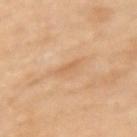patient — female, aged around 60 | acquisition — ~15 mm tile from a whole-body skin photo | location — the arm | size — about 2.5 mm | tile lighting — cross-polarized.